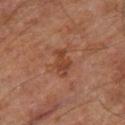workup = catalogued during a skin exam; not biopsied | anatomic site = the leg | image source = total-body-photography crop, ~15 mm field of view | patient = male, aged approximately 70.